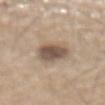Findings:
• lesion size · about 4.5 mm
• illumination · white-light
• image source · ~15 mm crop, total-body skin-cancer survey
• body site · the abdomen
• patient · male, aged 68–72